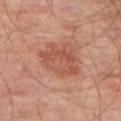Recorded during total-body skin imaging; not selected for excision or biopsy. A 15 mm crop from a total-body photograph taken for skin-cancer surveillance. Longest diameter approximately 5.5 mm. A male patient, in their 70s. From the left thigh.